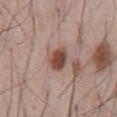follow-up = total-body-photography surveillance lesion; no biopsy
tile lighting = white-light illumination
patient = male, in their mid- to late 50s
size = ~3 mm (longest diameter)
image source = ~15 mm tile from a whole-body skin photo
anatomic site = the front of the torso
TBP lesion metrics = a lesion area of about 7 mm² and a shape eccentricity near 0.5